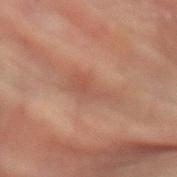This lesion was catalogued during total-body skin photography and was not selected for biopsy.
A roughly 15 mm field-of-view crop from a total-body skin photograph.
A female subject aged 78–82.
Longest diameter approximately 5.5 mm.
On the left forearm.
The lesion-visualizer software estimated border irregularity of about 5.5 on a 0–10 scale, internal color variation of about 3 on a 0–10 scale, and a peripheral color-asymmetry measure near 1. The software also gave an automated nevus-likeness rating near 0 out of 100 and a detector confidence of about 90 out of 100 that the crop contains a lesion.